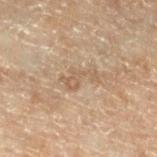Acquisition and patient details: Cropped from a total-body skin-imaging series; the visible field is about 15 mm. The lesion is located on the left lower leg. A male subject, aged around 70.